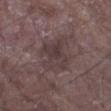Recorded during total-body skin imaging; not selected for excision or biopsy. Cropped from a whole-body photographic skin survey; the tile spans about 15 mm. Automated tile analysis of the lesion measured a shape eccentricity near 0.6. Located on the left forearm. A male patient aged approximately 65.